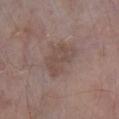Q: Was this lesion biopsied?
A: total-body-photography surveillance lesion; no biopsy
Q: Where on the body is the lesion?
A: the right lower leg
Q: What kind of image is this?
A: total-body-photography crop, ~15 mm field of view
Q: Patient demographics?
A: female, in their mid- to late 70s
Q: How was the tile lit?
A: white-light
Q: Automated lesion metrics?
A: a shape eccentricity near 0.8 and two-axis asymmetry of about 0.3; a border-irregularity index near 3.5/10, a color-variation rating of about 2/10, and a peripheral color-asymmetry measure near 0.5; a nevus-likeness score of about 0/100 and lesion-presence confidence of about 100/100